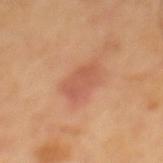Q: Was this lesion biopsied?
A: imaged on a skin check; not biopsied
Q: Lesion size?
A: about 4 mm
Q: What kind of image is this?
A: ~15 mm crop, total-body skin-cancer survey
Q: What are the patient's age and sex?
A: male, in their mid- to late 60s
Q: What lighting was used for the tile?
A: cross-polarized
Q: What did automated image analysis measure?
A: an average lesion color of about L≈55 a*≈26 b*≈33 (CIELAB), roughly 8 lightness units darker than nearby skin, and a normalized border contrast of about 5.5; a classifier nevus-likeness of about 10/100 and lesion-presence confidence of about 100/100
Q: Where on the body is the lesion?
A: the mid back